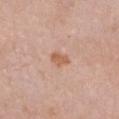Q: What did automated image analysis measure?
A: an area of roughly 3.5 mm² and a shape-asymmetry score of about 0.25 (0 = symmetric); an automated nevus-likeness rating near 60 out of 100 and a detector confidence of about 100 out of 100 that the crop contains a lesion
Q: What kind of image is this?
A: total-body-photography crop, ~15 mm field of view
Q: Lesion size?
A: about 2.5 mm
Q: What is the anatomic site?
A: the chest
Q: What are the patient's age and sex?
A: female, approximately 65 years of age